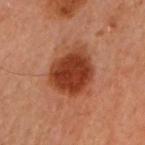The lesion was photographed on a routine skin check and not biopsied; there is no pathology result. Cropped from a total-body skin-imaging series; the visible field is about 15 mm. Automated image analysis of the tile measured an eccentricity of roughly 0.6 and a symmetry-axis asymmetry near 0.25. It also reported a border-irregularity index near 2.5/10 and a color-variation rating of about 3.5/10. The software also gave a nevus-likeness score of about 95/100. The lesion's longest dimension is about 6 mm. A female subject, aged approximately 60. This is a cross-polarized tile. On the left arm.